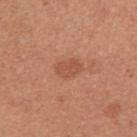follow-up — catalogued during a skin exam; not biopsied | acquisition — ~15 mm crop, total-body skin-cancer survey | body site — the right upper arm | subject — female, aged around 25.